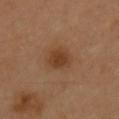Case summary:
- workup — imaged on a skin check; not biopsied
- location — the chest
- subject — male, approximately 40 years of age
- automated metrics — a lesion area of about 6 mm², a shape eccentricity near 0.55, and two-axis asymmetry of about 0.2; a lesion color around L≈39 a*≈20 b*≈33 in CIELAB, roughly 8 lightness units darker than nearby skin, and a normalized lesion–skin contrast near 7.5; a within-lesion color-variation index near 2.5/10 and radial color variation of about 0.5; a classifier nevus-likeness of about 85/100
- image source — ~15 mm tile from a whole-body skin photo
- illumination — cross-polarized illumination
- lesion diameter — ~3 mm (longest diameter)